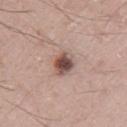Impression: Imaged during a routine full-body skin examination; the lesion was not biopsied and no histopathology is available. Clinical summary: Captured under white-light illumination. The lesion is located on the left upper arm. A male subject, aged around 25. A 15 mm close-up extracted from a 3D total-body photography capture. Longest diameter approximately 3 mm. Automated image analysis of the tile measured a lesion color around L≈50 a*≈19 b*≈23 in CIELAB, about 15 CIELAB-L* units darker than the surrounding skin, and a lesion-to-skin contrast of about 10 (normalized; higher = more distinct).